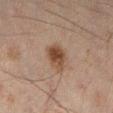follow-up: catalogued during a skin exam; not biopsied | location: the left lower leg | image source: 15 mm crop, total-body photography | subject: male, aged approximately 45.